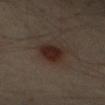Assessment: Captured during whole-body skin photography for melanoma surveillance; the lesion was not biopsied. Acquisition and patient details: Captured under cross-polarized illumination. A male subject, in their mid-60s. From the right upper arm. The lesion-visualizer software estimated an area of roughly 10 mm² and a symmetry-axis asymmetry near 0.25. The software also gave border irregularity of about 2.5 on a 0–10 scale, a within-lesion color-variation index near 3.5/10, and a peripheral color-asymmetry measure near 1. This image is a 15 mm lesion crop taken from a total-body photograph. Approximately 4.5 mm at its widest.